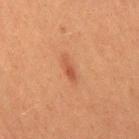| feature | finding |
|---|---|
| workup | catalogued during a skin exam; not biopsied |
| image-analysis metrics | a lesion color around L≈45 a*≈24 b*≈31 in CIELAB, a lesion–skin lightness drop of about 7, and a lesion-to-skin contrast of about 6 (normalized; higher = more distinct); a classifier nevus-likeness of about 70/100 and lesion-presence confidence of about 100/100 |
| size | ~3.5 mm (longest diameter) |
| lighting | cross-polarized illumination |
| subject | female, about 50 years old |
| acquisition | 15 mm crop, total-body photography |
| anatomic site | the right thigh |